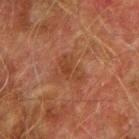Recorded during total-body skin imaging; not selected for excision or biopsy. On the right upper arm. This is a cross-polarized tile. An algorithmic analysis of the crop reported an outline eccentricity of about 0.75 (0 = round, 1 = elongated) and a symmetry-axis asymmetry near 0.45. It also reported an average lesion color of about L≈34 a*≈22 b*≈29 (CIELAB), roughly 6 lightness units darker than nearby skin, and a lesion-to-skin contrast of about 5.5 (normalized; higher = more distinct). The software also gave peripheral color asymmetry of about 0.5. The software also gave a nevus-likeness score of about 0/100 and a detector confidence of about 100 out of 100 that the crop contains a lesion. A roughly 15 mm field-of-view crop from a total-body skin photograph. Measured at roughly 3.5 mm in maximum diameter. A male subject in their mid- to late 70s.